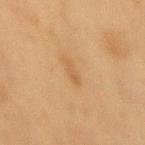This is a cross-polarized tile. A female subject aged approximately 40. A 15 mm close-up extracted from a 3D total-body photography capture. On the mid back. The recorded lesion diameter is about 3 mm.